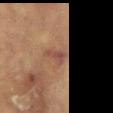Q: Was this lesion biopsied?
A: no biopsy performed (imaged during a skin exam)